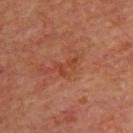Context: Automated image analysis of the tile measured an area of roughly 3 mm², a shape eccentricity near 0.85, and two-axis asymmetry of about 0.55. And it measured a color-variation rating of about 0/10 and peripheral color asymmetry of about 0. The subject is a male aged around 70. The tile uses cross-polarized illumination. The lesion is located on the upper back. Cropped from a total-body skin-imaging series; the visible field is about 15 mm. The recorded lesion diameter is about 2.5 mm.